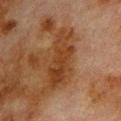A close-up tile cropped from a whole-body skin photograph, about 15 mm across. A male subject, aged 78 to 82. This is a cross-polarized tile. An algorithmic analysis of the crop reported an average lesion color of about L≈30 a*≈19 b*≈29 (CIELAB), about 7 CIELAB-L* units darker than the surrounding skin, and a normalized border contrast of about 7.5. It also reported a classifier nevus-likeness of about 0/100. The lesion is on the back. The recorded lesion diameter is about 7 mm.